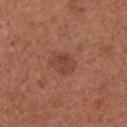workup=catalogued during a skin exam; not biopsied
image source=~15 mm tile from a whole-body skin photo
location=the right upper arm
subject=female, in their mid-60s